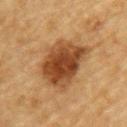Assessment: Imaged during a routine full-body skin examination; the lesion was not biopsied and no histopathology is available. Clinical summary: A 15 mm close-up extracted from a 3D total-body photography capture. Imaged with cross-polarized lighting. A male patient about 85 years old or older. From the back.